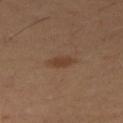workup: imaged on a skin check; not biopsied
patient: female, about 55 years old
imaging modality: total-body-photography crop, ~15 mm field of view
diameter: about 3.5 mm
location: the right thigh
illumination: cross-polarized illumination